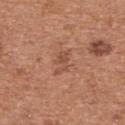* biopsy status — imaged on a skin check; not biopsied
* patient — female, aged around 60
* acquisition — total-body-photography crop, ~15 mm field of view
* illumination — white-light illumination
* anatomic site — the upper back
* diameter — ~2.5 mm (longest diameter)